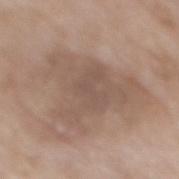Findings:
– notes — no biopsy performed (imaged during a skin exam)
– lighting — white-light
– location — the mid back
– subject — female, about 75 years old
– size — about 8.5 mm
– image source — ~15 mm tile from a whole-body skin photo